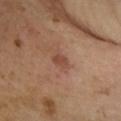follow-up: no biopsy performed (imaged during a skin exam)
patient: female, aged approximately 45
acquisition: ~15 mm tile from a whole-body skin photo
TBP lesion metrics: a footprint of about 2.5 mm² and a shape eccentricity near 0.85; an average lesion color of about L≈46 a*≈23 b*≈28 (CIELAB), a lesion–skin lightness drop of about 8, and a lesion-to-skin contrast of about 6.5 (normalized; higher = more distinct); a border-irregularity index near 2.5/10 and radial color variation of about 0
location: the right forearm
lesion diameter: ~2.5 mm (longest diameter)